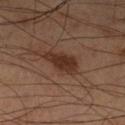The lesion was tiled from a total-body skin photograph and was not biopsied.
The subject is a male aged approximately 60.
On the leg.
Captured under cross-polarized illumination.
Cropped from a total-body skin-imaging series; the visible field is about 15 mm.
Approximately 4 mm at its widest.
Automated image analysis of the tile measured an average lesion color of about L≈31 a*≈19 b*≈25 (CIELAB), a lesion–skin lightness drop of about 10, and a normalized lesion–skin contrast near 9.5. And it measured a nevus-likeness score of about 90/100 and a detector confidence of about 100 out of 100 that the crop contains a lesion.